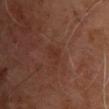biopsy_status: not biopsied; imaged during a skin examination
automated_metrics:
  cielab_L: 30
  cielab_a: 21
  cielab_b: 25
  vs_skin_contrast_norm: 4.5
  border_irregularity_0_10: 4.0
  color_variation_0_10: 1.0
  peripheral_color_asymmetry: 0.5
patient:
  sex: male
  age_approx: 65
image:
  source: total-body photography crop
  field_of_view_mm: 15
lesion_size:
  long_diameter_mm_approx: 2.5
lighting: cross-polarized
site: chest